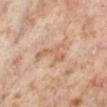<case>
<site>right lower leg</site>
<image>
  <source>total-body photography crop</source>
  <field_of_view_mm>15</field_of_view_mm>
</image>
<patient>
  <sex>female</sex>
  <age_approx>50</age_approx>
</patient>
<automated_metrics>
  <cielab_L>60</cielab_L>
  <cielab_a>20</cielab_a>
  <cielab_b>30</cielab_b>
  <vs_skin_darker_L>8.0</vs_skin_darker_L>
</automated_metrics>
</case>